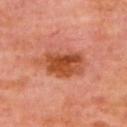Captured during whole-body skin photography for melanoma surveillance; the lesion was not biopsied.
The recorded lesion diameter is about 6 mm.
Imaged with cross-polarized lighting.
A region of skin cropped from a whole-body photographic capture, roughly 15 mm wide.
A female subject in their 50s.
Located on the upper back.